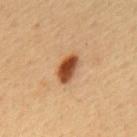| feature | finding |
|---|---|
| follow-up | catalogued during a skin exam; not biopsied |
| acquisition | ~15 mm tile from a whole-body skin photo |
| body site | the mid back |
| lesion size | ≈3.5 mm |
| subject | male, aged around 55 |
| lighting | cross-polarized |
| image-analysis metrics | a lesion color around L≈43 a*≈23 b*≈34 in CIELAB; a nevus-likeness score of about 100/100 and a detector confidence of about 100 out of 100 that the crop contains a lesion |